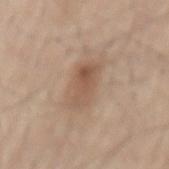Findings:
- notes: imaged on a skin check; not biopsied
- body site: the mid back
- imaging modality: ~15 mm tile from a whole-body skin photo
- lesion diameter: about 4 mm
- subject: male, aged approximately 65